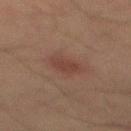Imaged during a routine full-body skin examination; the lesion was not biopsied and no histopathology is available.